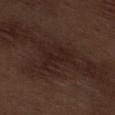Image and clinical context: The lesion is located on the right thigh. Automated tile analysis of the lesion measured border irregularity of about 7.5 on a 0–10 scale and a within-lesion color-variation index near 1.5/10. The analysis additionally found an automated nevus-likeness rating near 0 out of 100 and a lesion-detection confidence of about 95/100. The lesion's longest dimension is about 4 mm. A male subject, aged 68–72. Cropped from a whole-body photographic skin survey; the tile spans about 15 mm.